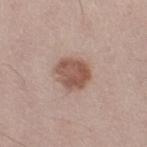{"biopsy_status": "not biopsied; imaged during a skin examination", "lesion_size": {"long_diameter_mm_approx": 4.0}, "automated_metrics": {"area_mm2_approx": 10.0, "border_irregularity_0_10": 2.0, "color_variation_0_10": 3.5, "peripheral_color_asymmetry": 1.0}, "patient": {"sex": "female", "age_approx": 40}, "site": "right thigh", "lighting": "white-light", "image": {"source": "total-body photography crop", "field_of_view_mm": 15}}